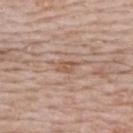notes = total-body-photography surveillance lesion; no biopsy
site = the upper back
subject = female, aged approximately 65
imaging modality = 15 mm crop, total-body photography